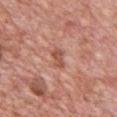Captured during whole-body skin photography for melanoma surveillance; the lesion was not biopsied. Imaged with white-light lighting. A male patient, approximately 50 years of age. From the chest. Automated image analysis of the tile measured an area of roughly 3.5 mm², an eccentricity of roughly 0.85, and a shape-asymmetry score of about 0.35 (0 = symmetric). And it measured a mean CIELAB color near L≈53 a*≈26 b*≈30 and roughly 10 lightness units darker than nearby skin. The analysis additionally found lesion-presence confidence of about 100/100. A region of skin cropped from a whole-body photographic capture, roughly 15 mm wide. Approximately 2.5 mm at its widest.